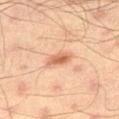Assessment:
Part of a total-body skin-imaging series; this lesion was reviewed on a skin check and was not flagged for biopsy.
Acquisition and patient details:
A lesion tile, about 15 mm wide, cut from a 3D total-body photograph. The lesion is on the leg. About 3.5 mm across. A male subject, aged 43–47. Automated tile analysis of the lesion measured an area of roughly 4.5 mm², an eccentricity of roughly 0.9, and a shape-asymmetry score of about 0.25 (0 = symmetric). The software also gave an average lesion color of about L≈52 a*≈21 b*≈30 (CIELAB), about 10 CIELAB-L* units darker than the surrounding skin, and a normalized lesion–skin contrast near 7.5.